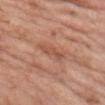notes: total-body-photography surveillance lesion; no biopsy
diameter: about 3.5 mm
anatomic site: the front of the torso
image source: 15 mm crop, total-body photography
subject: male, in their 60s
automated lesion analysis: a mean CIELAB color near L≈52 a*≈23 b*≈31, a lesion–skin lightness drop of about 7, and a normalized border contrast of about 5.5; a classifier nevus-likeness of about 0/100 and a lesion-detection confidence of about 95/100
tile lighting: white-light